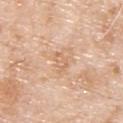notes = no biopsy performed (imaged during a skin exam); image = 15 mm crop, total-body photography; subject = male, aged 78 to 82; site = the upper back; lighting = white-light; lesion diameter = about 2.5 mm.